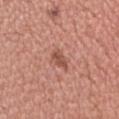notes: catalogued during a skin exam; not biopsied | lighting: white-light illumination | patient: male, aged 33–37 | anatomic site: the head or neck | image-analysis metrics: a lesion color around L≈52 a*≈26 b*≈29 in CIELAB, roughly 10 lightness units darker than nearby skin, and a normalized lesion–skin contrast near 6.5; an automated nevus-likeness rating near 20 out of 100 and lesion-presence confidence of about 100/100 | acquisition: total-body-photography crop, ~15 mm field of view.